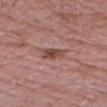Impression: The lesion was photographed on a routine skin check and not biopsied; there is no pathology result. Context: The lesion is on the head or neck. The lesion's longest dimension is about 3 mm. A roughly 15 mm field-of-view crop from a total-body skin photograph. Automated image analysis of the tile measured a lesion area of about 5 mm², an eccentricity of roughly 0.8, and two-axis asymmetry of about 0.25. This is a white-light tile. A male subject, aged 48–52.